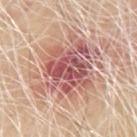follow-up = imaged on a skin check; not biopsied
lesion size = ~8 mm (longest diameter)
image = 15 mm crop, total-body photography
body site = the arm
subject = male, aged around 65
lighting = white-light